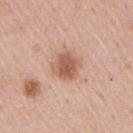Imaged during a routine full-body skin examination; the lesion was not biopsied and no histopathology is available.
A male subject aged around 60.
The recorded lesion diameter is about 3.5 mm.
On the right upper arm.
A close-up tile cropped from a whole-body skin photograph, about 15 mm across.